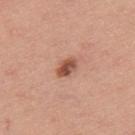The lesion was tiled from a total-body skin photograph and was not biopsied.
The lesion's longest dimension is about 3 mm.
The lesion is located on the upper back.
A male patient, roughly 40 years of age.
The lesion-visualizer software estimated a footprint of about 4 mm², an outline eccentricity of about 0.8 (0 = round, 1 = elongated), and a shape-asymmetry score of about 0.25 (0 = symmetric). The analysis additionally found a border-irregularity rating of about 2/10, internal color variation of about 5 on a 0–10 scale, and peripheral color asymmetry of about 1.5. The analysis additionally found a classifier nevus-likeness of about 85/100 and lesion-presence confidence of about 100/100.
This image is a 15 mm lesion crop taken from a total-body photograph.
Captured under white-light illumination.